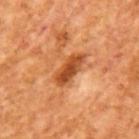The lesion was tiled from a total-body skin photograph and was not biopsied. A close-up tile cropped from a whole-body skin photograph, about 15 mm across. A male patient in their mid-60s.